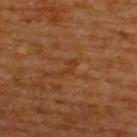* workup · total-body-photography surveillance lesion; no biopsy
* subject · male, aged 63 to 67
* size · ~2.5 mm (longest diameter)
* lighting · cross-polarized
* location · the upper back
* acquisition · total-body-photography crop, ~15 mm field of view